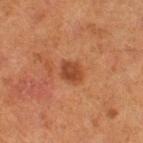Imaged during a routine full-body skin examination; the lesion was not biopsied and no histopathology is available.
The subject is a female approximately 50 years of age.
The lesion-visualizer software estimated a lesion area of about 4.5 mm², an outline eccentricity of about 0.6 (0 = round, 1 = elongated), and a symmetry-axis asymmetry near 0.2. The analysis additionally found border irregularity of about 1.5 on a 0–10 scale, a color-variation rating of about 2/10, and a peripheral color-asymmetry measure near 1.
On the right thigh.
Longest diameter approximately 3 mm.
A close-up tile cropped from a whole-body skin photograph, about 15 mm across.
Captured under cross-polarized illumination.